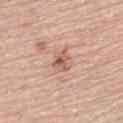notes: total-body-photography surveillance lesion; no biopsy
acquisition: ~15 mm crop, total-body skin-cancer survey
tile lighting: white-light illumination
TBP lesion metrics: a shape eccentricity near 0.7 and two-axis asymmetry of about 0.3; a mean CIELAB color near L≈59 a*≈22 b*≈29 and a lesion–skin lightness drop of about 11; a color-variation rating of about 6.5/10 and a peripheral color-asymmetry measure near 2; a nevus-likeness score of about 0/100 and lesion-presence confidence of about 100/100
location: the left upper arm
lesion size: ≈3 mm
subject: female, aged 63–67